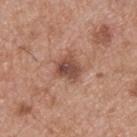| key | value |
|---|---|
| biopsy status | total-body-photography surveillance lesion; no biopsy |
| automated metrics | a footprint of about 7.5 mm², a shape eccentricity near 0.5, and a symmetry-axis asymmetry near 0.35; a border-irregularity rating of about 3.5/10, a within-lesion color-variation index near 5/10, and peripheral color asymmetry of about 1.5 |
| lesion diameter | ~3.5 mm (longest diameter) |
| subject | male, approximately 55 years of age |
| image | total-body-photography crop, ~15 mm field of view |
| anatomic site | the upper back |